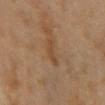Impression:
Imaged during a routine full-body skin examination; the lesion was not biopsied and no histopathology is available.
Context:
An algorithmic analysis of the crop reported an average lesion color of about L≈44 a*≈18 b*≈32 (CIELAB), roughly 6 lightness units darker than nearby skin, and a normalized border contrast of about 5.5. A female patient, about 35 years old. From the chest. Captured under cross-polarized illumination. About 3.5 mm across. A 15 mm close-up tile from a total-body photography series done for melanoma screening.